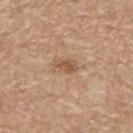Impression:
Captured during whole-body skin photography for melanoma surveillance; the lesion was not biopsied.
Context:
A lesion tile, about 15 mm wide, cut from a 3D total-body photograph. Automated image analysis of the tile measured a mean CIELAB color near L≈55 a*≈19 b*≈33, about 10 CIELAB-L* units darker than the surrounding skin, and a normalized border contrast of about 7. It also reported a nevus-likeness score of about 55/100 and a detector confidence of about 100 out of 100 that the crop contains a lesion. The lesion is located on the upper back. Longest diameter approximately 3 mm. Imaged with white-light lighting. A male patient, in their 70s.